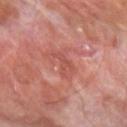{"biopsy_status": "not biopsied; imaged during a skin examination", "image": {"source": "total-body photography crop", "field_of_view_mm": 15}, "lesion_size": {"long_diameter_mm_approx": 3.0}, "automated_metrics": {"cielab_L": 49, "cielab_a": 29, "cielab_b": 27, "vs_skin_darker_L": 7.0, "vs_skin_contrast_norm": 5.0, "nevus_likeness_0_100": 0, "lesion_detection_confidence_0_100": 100}, "site": "arm", "patient": {"sex": "male", "age_approx": 65}, "lighting": "cross-polarized"}